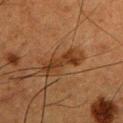biopsy status: imaged on a skin check; not biopsied
acquisition: ~15 mm crop, total-body skin-cancer survey
illumination: cross-polarized
subject: male, approximately 50 years of age
location: the left upper arm
image-analysis metrics: a lesion area of about 7 mm² and a shape-asymmetry score of about 0.3 (0 = symmetric); a border-irregularity index near 5/10 and internal color variation of about 3 on a 0–10 scale; a lesion-detection confidence of about 100/100